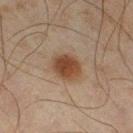This lesion was catalogued during total-body skin photography and was not selected for biopsy. A male patient, roughly 45 years of age. The tile uses cross-polarized illumination. The lesion's longest dimension is about 3.5 mm. Located on the right thigh. Cropped from a whole-body photographic skin survey; the tile spans about 15 mm.